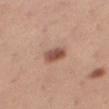{
  "biopsy_status": "not biopsied; imaged during a skin examination",
  "lighting": "white-light",
  "patient": {
    "sex": "female",
    "age_approx": 25
  },
  "site": "leg",
  "image": {
    "source": "total-body photography crop",
    "field_of_view_mm": 15
  }
}